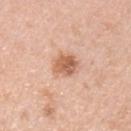Part of a total-body skin-imaging series; this lesion was reviewed on a skin check and was not flagged for biopsy.
This is a white-light tile.
A region of skin cropped from a whole-body photographic capture, roughly 15 mm wide.
The total-body-photography lesion software estimated a border-irregularity rating of about 2.5/10, a color-variation rating of about 4/10, and radial color variation of about 1.5.
A male subject, aged around 45.
Longest diameter approximately 3 mm.
On the chest.